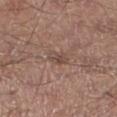Impression:
Recorded during total-body skin imaging; not selected for excision or biopsy.
Clinical summary:
Imaged with white-light lighting. A lesion tile, about 15 mm wide, cut from a 3D total-body photograph. Measured at roughly 3 mm in maximum diameter. On the left lower leg. A male patient aged 53 to 57.